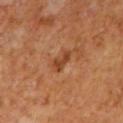Q: Was this lesion biopsied?
A: catalogued during a skin exam; not biopsied
Q: What are the patient's age and sex?
A: male, in their mid- to late 60s
Q: Where on the body is the lesion?
A: the mid back
Q: What is the imaging modality?
A: ~15 mm crop, total-body skin-cancer survey
Q: Automated lesion metrics?
A: a lesion color around L≈41 a*≈25 b*≈35 in CIELAB, a lesion–skin lightness drop of about 9, and a lesion-to-skin contrast of about 7.5 (normalized; higher = more distinct)